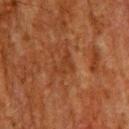<record>
  <biopsy_status>not biopsied; imaged during a skin examination</biopsy_status>
  <image>
    <source>total-body photography crop</source>
    <field_of_view_mm>15</field_of_view_mm>
  </image>
  <automated_metrics>
    <area_mm2_approx>3.5</area_mm2_approx>
    <eccentricity>0.75</eccentricity>
    <cielab_L>29</cielab_L>
    <cielab_a>21</cielab_a>
    <cielab_b>29</cielab_b>
    <vs_skin_darker_L>5.0</vs_skin_darker_L>
    <vs_skin_contrast_norm>5.0</vs_skin_contrast_norm>
    <border_irregularity_0_10>7.0</border_irregularity_0_10>
    <color_variation_0_10>0.0</color_variation_0_10>
    <peripheral_color_asymmetry>0.0</peripheral_color_asymmetry>
  </automated_metrics>
  <site>back</site>
  <lesion_size>
    <long_diameter_mm_approx>3.0</long_diameter_mm_approx>
  </lesion_size>
  <lighting>cross-polarized</lighting>
  <patient>
    <sex>male</sex>
    <age_approx>60</age_approx>
  </patient>
</record>